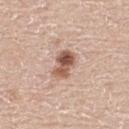Q: Was this lesion biopsied?
A: catalogued during a skin exam; not biopsied
Q: How was this image acquired?
A: total-body-photography crop, ~15 mm field of view
Q: How large is the lesion?
A: about 3.5 mm
Q: What lighting was used for the tile?
A: white-light illumination
Q: What is the anatomic site?
A: the upper back
Q: Patient demographics?
A: male, aged 58 to 62